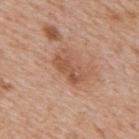Notes:
* notes: no biopsy performed (imaged during a skin exam)
* anatomic site: the mid back
* size: ≈4 mm
* image source: ~15 mm tile from a whole-body skin photo
* subject: male, aged 63–67
* automated metrics: border irregularity of about 3.5 on a 0–10 scale, a color-variation rating of about 3/10, and a peripheral color-asymmetry measure near 1
* lighting: white-light illumination